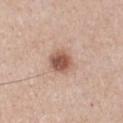<tbp_lesion>
  <patient>
    <sex>male</sex>
    <age_approx>60</age_approx>
  </patient>
  <image>
    <source>total-body photography crop</source>
    <field_of_view_mm>15</field_of_view_mm>
  </image>
  <site>chest</site>
  <lesion_size>
    <long_diameter_mm_approx>3.0</long_diameter_mm_approx>
  </lesion_size>
  <lighting>white-light</lighting>
  <automated_metrics>
    <vs_skin_contrast_norm>9.5</vs_skin_contrast_norm>
    <color_variation_0_10>3.5</color_variation_0_10>
    <peripheral_color_asymmetry>1.0</peripheral_color_asymmetry>
    <lesion_detection_confidence_0_100>100</lesion_detection_confidence_0_100>
  </automated_metrics>
</tbp_lesion>